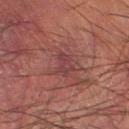Clinical impression:
Captured during whole-body skin photography for melanoma surveillance; the lesion was not biopsied.
Image and clinical context:
A male subject aged 48 to 52. The tile uses cross-polarized illumination. The lesion is on the left lower leg. A 15 mm close-up tile from a total-body photography series done for melanoma screening. Automated image analysis of the tile measured a mean CIELAB color near L≈30 a*≈20 b*≈16, roughly 5 lightness units darker than nearby skin, and a normalized lesion–skin contrast near 5.5. The software also gave a border-irregularity rating of about 3/10 and a peripheral color-asymmetry measure near 1.5. And it measured a nevus-likeness score of about 0/100.